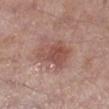acquisition: ~15 mm tile from a whole-body skin photo
patient: male, aged 53 to 57
site: the right lower leg